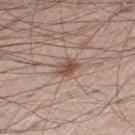<case>
<biopsy_status>not biopsied; imaged during a skin examination</biopsy_status>
<patient>
  <sex>male</sex>
  <age_approx>70</age_approx>
</patient>
<site>left leg</site>
<image>
  <source>total-body photography crop</source>
  <field_of_view_mm>15</field_of_view_mm>
</image>
</case>